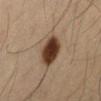| field | value |
|---|---|
| follow-up | no biopsy performed (imaged during a skin exam) |
| image | total-body-photography crop, ~15 mm field of view |
| site | the front of the torso |
| subject | male, aged approximately 35 |
| illumination | cross-polarized |